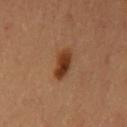Case summary:
* biopsy status — total-body-photography surveillance lesion; no biopsy
* subject — female, aged 33–37
* image — total-body-photography crop, ~15 mm field of view
* site — the left upper arm